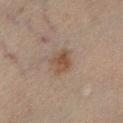Recorded during total-body skin imaging; not selected for excision or biopsy.
On the left lower leg.
A male subject about 45 years old.
Cropped from a total-body skin-imaging series; the visible field is about 15 mm.